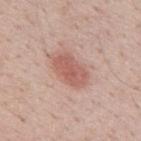  biopsy_status: not biopsied; imaged during a skin examination
  image:
    source: total-body photography crop
    field_of_view_mm: 15
  lesion_size:
    long_diameter_mm_approx: 4.5
  lighting: white-light
  site: back
  patient:
    sex: male
    age_approx: 40